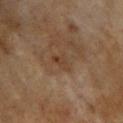<tbp_lesion>
<automated_metrics>
  <peripheral_color_asymmetry>0.5</peripheral_color_asymmetry>
  <lesion_detection_confidence_0_100>100</lesion_detection_confidence_0_100>
</automated_metrics>
<patient>
  <sex>female</sex>
  <age_approx>60</age_approx>
</patient>
<site>front of the torso</site>
<image>
  <source>total-body photography crop</source>
  <field_of_view_mm>15</field_of_view_mm>
</image>
</tbp_lesion>